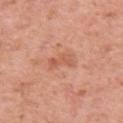The lesion was tiled from a total-body skin photograph and was not biopsied.